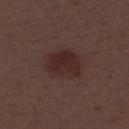Recorded during total-body skin imaging; not selected for excision or biopsy.